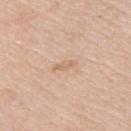Image and clinical context: The subject is a female in their mid-50s. An algorithmic analysis of the crop reported a lesion area of about 3 mm², an outline eccentricity of about 0.9 (0 = round, 1 = elongated), and two-axis asymmetry of about 0.3. And it measured a mean CIELAB color near L≈67 a*≈17 b*≈34, about 7 CIELAB-L* units darker than the surrounding skin, and a lesion-to-skin contrast of about 5 (normalized; higher = more distinct). It also reported a border-irregularity index near 3.5/10 and internal color variation of about 0.5 on a 0–10 scale. Longest diameter approximately 3 mm. This image is a 15 mm lesion crop taken from a total-body photograph. From the upper back. This is a white-light tile.